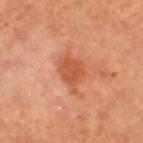{"biopsy_status": "not biopsied; imaged during a skin examination", "image": {"source": "total-body photography crop", "field_of_view_mm": 15}, "patient": {"age_approx": 70}, "site": "leg"}